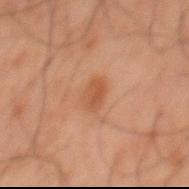The lesion was tiled from a total-body skin photograph and was not biopsied. The lesion is located on the mid back. This image is a 15 mm lesion crop taken from a total-body photograph. A male subject, aged 58–62. Approximately 3 mm at its widest.